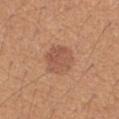Imaged during a routine full-body skin examination; the lesion was not biopsied and no histopathology is available. A female subject, aged around 40. The lesion is on the left forearm. Cropped from a whole-body photographic skin survey; the tile spans about 15 mm.